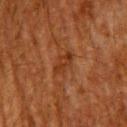workup = total-body-photography surveillance lesion; no biopsy | lighting = cross-polarized illumination | anatomic site = the upper back | lesion diameter = about 3 mm | patient = male, aged 58–62 | acquisition = total-body-photography crop, ~15 mm field of view.